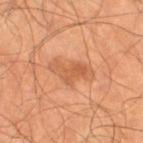Impression:
The lesion was photographed on a routine skin check and not biopsied; there is no pathology result.
Background:
Cropped from a total-body skin-imaging series; the visible field is about 15 mm. The lesion is located on the left thigh. A male subject, in their mid-60s. This is a cross-polarized tile.